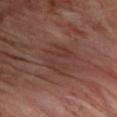Impression: The lesion was photographed on a routine skin check and not biopsied; there is no pathology result. Background: From the chest. This is a cross-polarized tile. Longest diameter approximately 4 mm. A 15 mm close-up tile from a total-body photography series done for melanoma screening. The patient is a male approximately 65 years of age.